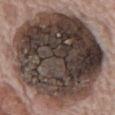Q: Is there a histopathology result?
A: no biopsy performed (imaged during a skin exam)
Q: Patient demographics?
A: male, roughly 70 years of age
Q: How was the tile lit?
A: white-light illumination
Q: What is the imaging modality?
A: ~15 mm crop, total-body skin-cancer survey
Q: What is the anatomic site?
A: the abdomen
Q: Lesion size?
A: ≈13.5 mm